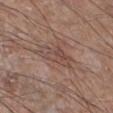The lesion was tiled from a total-body skin photograph and was not biopsied.
From the right lower leg.
The patient is a male in their mid- to late 60s.
Imaged with white-light lighting.
Automated image analysis of the tile measured an eccentricity of roughly 0.9. It also reported a border-irregularity rating of about 5.5/10, internal color variation of about 4.5 on a 0–10 scale, and radial color variation of about 2.
A region of skin cropped from a whole-body photographic capture, roughly 15 mm wide.
Approximately 5 mm at its widest.